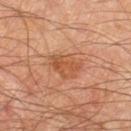biopsy status = no biopsy performed (imaged during a skin exam); patient = male, aged 68–72; tile lighting = cross-polarized illumination; lesion size = ≈4 mm; acquisition = ~15 mm crop, total-body skin-cancer survey; body site = the right lower leg.